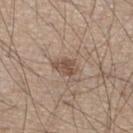Recorded during total-body skin imaging; not selected for excision or biopsy. Captured under white-light illumination. About 3 mm across. A close-up tile cropped from a whole-body skin photograph, about 15 mm across. A male patient, approximately 45 years of age. An algorithmic analysis of the crop reported a mean CIELAB color near L≈50 a*≈16 b*≈26, about 10 CIELAB-L* units darker than the surrounding skin, and a normalized lesion–skin contrast near 7.5. The analysis additionally found border irregularity of about 2.5 on a 0–10 scale and a color-variation rating of about 2.5/10. From the right lower leg.